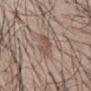- biopsy status — no biopsy performed (imaged during a skin exam)
- lesion size — ~4 mm (longest diameter)
- subject — male, aged around 45
- image source — ~15 mm crop, total-body skin-cancer survey
- anatomic site — the mid back